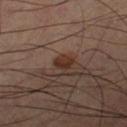Recorded during total-body skin imaging; not selected for excision or biopsy. A male subject, in their 60s. The lesion is located on the right thigh. Cropped from a whole-body photographic skin survey; the tile spans about 15 mm. Longest diameter approximately 2 mm.